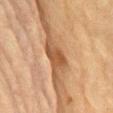The lesion is located on the mid back. Automated tile analysis of the lesion measured an average lesion color of about L≈44 a*≈21 b*≈33 (CIELAB), roughly 11 lightness units darker than nearby skin, and a lesion-to-skin contrast of about 8.5 (normalized; higher = more distinct). And it measured an automated nevus-likeness rating near 25 out of 100 and a lesion-detection confidence of about 100/100. This is a cross-polarized tile. About 3 mm across. Cropped from a total-body skin-imaging series; the visible field is about 15 mm. A female patient aged approximately 80.